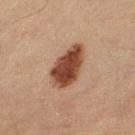patient = male, in their 60s
body site = the left lower leg
imaging modality = 15 mm crop, total-body photography
automated metrics = a lesion color around L≈36 a*≈20 b*≈26 in CIELAB and a normalized lesion–skin contrast near 13; border irregularity of about 2.5 on a 0–10 scale and a peripheral color-asymmetry measure near 1.5; an automated nevus-likeness rating near 100 out of 100 and lesion-presence confidence of about 100/100
illumination = cross-polarized